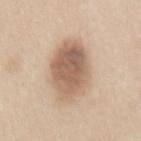{"biopsy_status": "not biopsied; imaged during a skin examination", "patient": {"sex": "female", "age_approx": 40}, "lighting": "white-light", "image": {"source": "total-body photography crop", "field_of_view_mm": 15}, "lesion_size": {"long_diameter_mm_approx": 7.5}, "site": "back"}